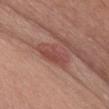Part of a total-body skin-imaging series; this lesion was reviewed on a skin check and was not flagged for biopsy. Automated tile analysis of the lesion measured a mean CIELAB color near L≈48 a*≈25 b*≈25, a lesion–skin lightness drop of about 9, and a normalized lesion–skin contrast near 7. And it measured a border-irregularity index near 3/10, a within-lesion color-variation index near 3/10, and a peripheral color-asymmetry measure near 1. The software also gave a nevus-likeness score of about 45/100 and a detector confidence of about 100 out of 100 that the crop contains a lesion. About 5 mm across. The tile uses white-light illumination. A close-up tile cropped from a whole-body skin photograph, about 15 mm across. Located on the chest. A female patient, aged 48–52.